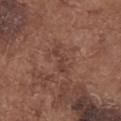Background: From the upper back. The recorded lesion diameter is about 3.5 mm. This is a white-light tile. A 15 mm crop from a total-body photograph taken for skin-cancer surveillance. A female subject, roughly 75 years of age.